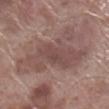Q: Was this lesion biopsied?
A: total-body-photography surveillance lesion; no biopsy
Q: What is the anatomic site?
A: the right lower leg
Q: How was this image acquired?
A: total-body-photography crop, ~15 mm field of view
Q: Who is the patient?
A: male, in their 70s
Q: Lesion size?
A: about 9.5 mm
Q: What did automated image analysis measure?
A: a lesion color around L≈48 a*≈18 b*≈20 in CIELAB, roughly 8 lightness units darker than nearby skin, and a lesion-to-skin contrast of about 6.5 (normalized; higher = more distinct); peripheral color asymmetry of about 1